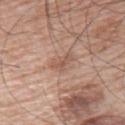Clinical impression: Imaged during a routine full-body skin examination; the lesion was not biopsied and no histopathology is available. Context: The recorded lesion diameter is about 3 mm. The lesion is on the upper back. A male subject, in their mid- to late 60s. A roughly 15 mm field-of-view crop from a total-body skin photograph. This is a white-light tile. Automated tile analysis of the lesion measured about 8 CIELAB-L* units darker than the surrounding skin and a normalized lesion–skin contrast near 5.5. The software also gave a border-irregularity index near 4.5/10 and internal color variation of about 1 on a 0–10 scale.